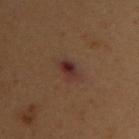<lesion>
<biopsy_status>not biopsied; imaged during a skin examination</biopsy_status>
<patient>
  <sex>female</sex>
  <age_approx>50</age_approx>
</patient>
<image>
  <source>total-body photography crop</source>
  <field_of_view_mm>15</field_of_view_mm>
</image>
<site>chest</site>
<lesion_size>
  <long_diameter_mm_approx>3.0</long_diameter_mm_approx>
</lesion_size>
</lesion>